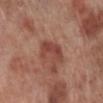Assessment: The lesion was photographed on a routine skin check and not biopsied; there is no pathology result. Context: The patient is a male aged 68–72. A 15 mm crop from a total-body photograph taken for skin-cancer surveillance. The recorded lesion diameter is about 4 mm. The lesion is on the right lower leg.